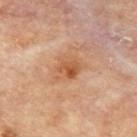follow-up: no biopsy performed (imaged during a skin exam)
patient: male, aged approximately 85 or older
image source: ~15 mm tile from a whole-body skin photo
image-analysis metrics: a lesion area of about 8 mm², an eccentricity of roughly 0.65, and a shape-asymmetry score of about 0.25 (0 = symmetric); an average lesion color of about L≈55 a*≈23 b*≈36 (CIELAB), a lesion–skin lightness drop of about 8, and a lesion-to-skin contrast of about 6.5 (normalized; higher = more distinct); a border-irregularity rating of about 3/10, a color-variation rating of about 6/10, and a peripheral color-asymmetry measure near 2; a classifier nevus-likeness of about 15/100 and a lesion-detection confidence of about 100/100
lesion diameter: about 4 mm
location: the back
lighting: cross-polarized